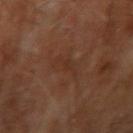Clinical impression: The lesion was photographed on a routine skin check and not biopsied; there is no pathology result. Image and clinical context: The tile uses cross-polarized illumination. A male subject roughly 70 years of age. Located on the arm. The recorded lesion diameter is about 5 mm. The lesion-visualizer software estimated a border-irregularity rating of about 5/10, internal color variation of about 2 on a 0–10 scale, and radial color variation of about 0.5. This image is a 15 mm lesion crop taken from a total-body photograph.